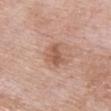This lesion was catalogued during total-body skin photography and was not selected for biopsy.
The lesion-visualizer software estimated a border-irregularity index near 3.5/10, internal color variation of about 2.5 on a 0–10 scale, and radial color variation of about 1. And it measured a nevus-likeness score of about 15/100 and a detector confidence of about 100 out of 100 that the crop contains a lesion.
About 3 mm across.
A female subject, aged 68–72.
The lesion is located on the front of the torso.
A 15 mm close-up tile from a total-body photography series done for melanoma screening.
Imaged with white-light lighting.